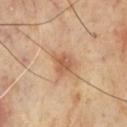Impression: The lesion was tiled from a total-body skin photograph and was not biopsied. Acquisition and patient details: The lesion's longest dimension is about 3 mm. The lesion is on the front of the torso. This image is a 15 mm lesion crop taken from a total-body photograph. The patient is a male aged 68 to 72.